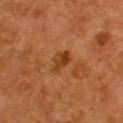Assessment:
Recorded during total-body skin imaging; not selected for excision or biopsy.
Clinical summary:
A male subject, in their mid-50s. From the back. Measured at roughly 3 mm in maximum diameter. An algorithmic analysis of the crop reported a border-irregularity index near 3/10 and a color-variation rating of about 4/10. The analysis additionally found a nevus-likeness score of about 40/100. A 15 mm crop from a total-body photograph taken for skin-cancer surveillance.